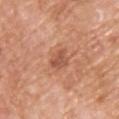| key | value |
|---|---|
| imaging modality | ~15 mm crop, total-body skin-cancer survey |
| lighting | white-light |
| anatomic site | the chest |
| lesion size | about 3.5 mm |
| subject | male, aged 58–62 |